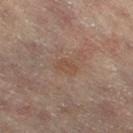<tbp_lesion>
  <biopsy_status>not biopsied; imaged during a skin examination</biopsy_status>
  <lighting>cross-polarized</lighting>
  <image>
    <source>total-body photography crop</source>
    <field_of_view_mm>15</field_of_view_mm>
  </image>
  <site>left leg</site>
  <patient>
    <sex>female</sex>
    <age_approx>80</age_approx>
  </patient>
  <lesion_size>
    <long_diameter_mm_approx>2.5</long_diameter_mm_approx>
  </lesion_size>
  <automated_metrics>
    <area_mm2_approx>4.0</area_mm2_approx>
    <eccentricity>0.75</eccentricity>
    <peripheral_color_asymmetry>0.5</peripheral_color_asymmetry>
  </automated_metrics>
</tbp_lesion>